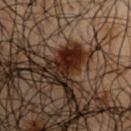Part of a total-body skin-imaging series; this lesion was reviewed on a skin check and was not flagged for biopsy.
This image is a 15 mm lesion crop taken from a total-body photograph.
The subject is a male aged around 50.
The lesion is on the right upper arm.